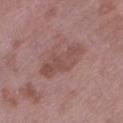Clinical impression: The lesion was tiled from a total-body skin photograph and was not biopsied. Acquisition and patient details: A region of skin cropped from a whole-body photographic capture, roughly 15 mm wide. The lesion's longest dimension is about 6 mm. The total-body-photography lesion software estimated a lesion–skin lightness drop of about 8 and a normalized lesion–skin contrast near 6. The software also gave a nevus-likeness score of about 0/100. A female subject, in their 70s. From the left lower leg. The tile uses white-light illumination.